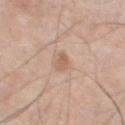Clinical impression: Part of a total-body skin-imaging series; this lesion was reviewed on a skin check and was not flagged for biopsy. Image and clinical context: Automated image analysis of the tile measured a border-irregularity rating of about 2.5/10, a color-variation rating of about 1/10, and peripheral color asymmetry of about 0. The recorded lesion diameter is about 2.5 mm. A close-up tile cropped from a whole-body skin photograph, about 15 mm across. A male subject, aged around 70. From the leg.